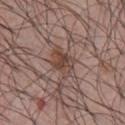Background: From the chest. A 15 mm close-up extracted from a 3D total-body photography capture. This is a white-light tile. The subject is a male aged around 40.